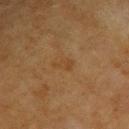A 15 mm close-up extracted from a 3D total-body photography capture.
Located on the right upper arm.
Approximately 2.5 mm at its widest.
A female subject, aged approximately 55.